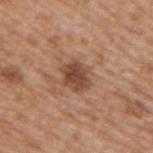The lesion was photographed on a routine skin check and not biopsied; there is no pathology result. An algorithmic analysis of the crop reported an area of roughly 7.5 mm², a shape eccentricity near 0.65, and a shape-asymmetry score of about 0.2 (0 = symmetric). The software also gave a border-irregularity index near 2/10, a color-variation rating of about 4/10, and radial color variation of about 1. It also reported a classifier nevus-likeness of about 70/100. Located on the right upper arm. Cropped from a whole-body photographic skin survey; the tile spans about 15 mm. Longest diameter approximately 3.5 mm. A male subject aged 63 to 67. Imaged with white-light lighting.